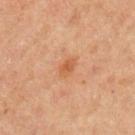Captured during whole-body skin photography for melanoma surveillance; the lesion was not biopsied.
This image is a 15 mm lesion crop taken from a total-body photograph.
About 2.5 mm across.
A male patient aged approximately 60.
Captured under cross-polarized illumination.
The lesion is on the arm.